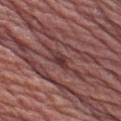  biopsy_status: not biopsied; imaged during a skin examination
  image:
    source: total-body photography crop
    field_of_view_mm: 15
  automated_metrics:
    cielab_L: 36
    cielab_a: 24
    cielab_b: 19
    vs_skin_darker_L: 8.0
    vs_skin_contrast_norm: 7.5
  lesion_size:
    long_diameter_mm_approx: 3.0
  lighting: white-light
  patient:
    sex: male
    age_approx: 75
  site: left upper arm